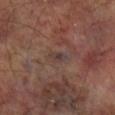{
  "biopsy_status": "not biopsied; imaged during a skin examination"
}